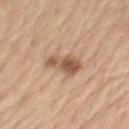Case summary:
– patient: male, aged approximately 70
– image: ~15 mm tile from a whole-body skin photo
– lesion diameter: ~5 mm (longest diameter)
– site: the left upper arm
– illumination: white-light